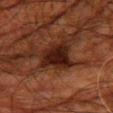anatomic site: the right thigh | subject: male, aged 78 to 82 | image: total-body-photography crop, ~15 mm field of view.